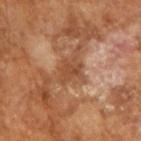notes: no biopsy performed (imaged during a skin exam)
lesion diameter: ≈3 mm
patient: male, about 65 years old
TBP lesion metrics: a footprint of about 5 mm², an eccentricity of roughly 0.55, and a shape-asymmetry score of about 0.35 (0 = symmetric); a lesion–skin lightness drop of about 9 and a lesion-to-skin contrast of about 7 (normalized; higher = more distinct); a border-irregularity rating of about 4/10, a color-variation rating of about 2/10, and a peripheral color-asymmetry measure near 0.5
image: ~15 mm crop, total-body skin-cancer survey